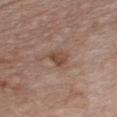Q: Was a biopsy performed?
A: total-body-photography surveillance lesion; no biopsy
Q: Illumination type?
A: white-light
Q: How was this image acquired?
A: ~15 mm crop, total-body skin-cancer survey
Q: Lesion location?
A: the chest
Q: Lesion size?
A: ≈3 mm
Q: Who is the patient?
A: male, approximately 55 years of age